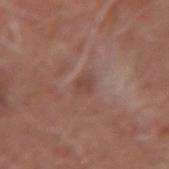workup: imaged on a skin check; not biopsied
tile lighting: white-light illumination
image-analysis metrics: a mean CIELAB color near L≈45 a*≈20 b*≈25 and a normalized border contrast of about 5.5; internal color variation of about 1 on a 0–10 scale and a peripheral color-asymmetry measure near 0.5
location: the left forearm
image: ~15 mm crop, total-body skin-cancer survey
diameter: about 2.5 mm
patient: male, aged approximately 65